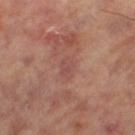Part of a total-body skin-imaging series; this lesion was reviewed on a skin check and was not flagged for biopsy. On the right leg. A female patient, aged approximately 65. Longest diameter approximately 2.5 mm. A 15 mm crop from a total-body photograph taken for skin-cancer surveillance. The tile uses cross-polarized illumination.